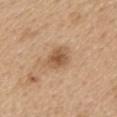| key | value |
|---|---|
| notes | catalogued during a skin exam; not biopsied |
| site | the upper back |
| subject | female, roughly 60 years of age |
| lesion diameter | about 3 mm |
| image-analysis metrics | a lesion area of about 6 mm², an outline eccentricity of about 0.55 (0 = round, 1 = elongated), and a shape-asymmetry score of about 0.2 (0 = symmetric); a nevus-likeness score of about 55/100 and a lesion-detection confidence of about 100/100 |
| lighting | white-light |
| image source | 15 mm crop, total-body photography |